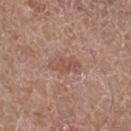Assessment:
Captured during whole-body skin photography for melanoma surveillance; the lesion was not biopsied.
Context:
The lesion is on the left forearm. A lesion tile, about 15 mm wide, cut from a 3D total-body photograph. A male subject approximately 60 years of age. Imaged with white-light lighting. The lesion-visualizer software estimated a lesion color around L≈52 a*≈21 b*≈26 in CIELAB, a lesion–skin lightness drop of about 7, and a normalized lesion–skin contrast near 5.5. It also reported a classifier nevus-likeness of about 0/100 and a lesion-detection confidence of about 100/100.